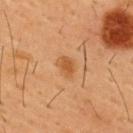{"biopsy_status": "not biopsied; imaged during a skin examination", "site": "chest", "patient": {"sex": "male", "age_approx": 55}, "image": {"source": "total-body photography crop", "field_of_view_mm": 15}}